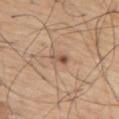biopsy_status: not biopsied; imaged during a skin examination
patient:
  sex: male
  age_approx: 75
image:
  source: total-body photography crop
  field_of_view_mm: 15
site: upper back